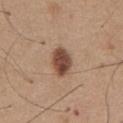Imaged during a routine full-body skin examination; the lesion was not biopsied and no histopathology is available.
From the abdomen.
Captured under white-light illumination.
This image is a 15 mm lesion crop taken from a total-body photograph.
A male subject, aged 63–67.
The lesion-visualizer software estimated a lesion–skin lightness drop of about 16 and a normalized border contrast of about 11.5. The analysis additionally found a border-irregularity index near 2/10 and peripheral color asymmetry of about 1.5.